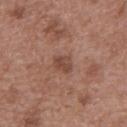This lesion was catalogued during total-body skin photography and was not selected for biopsy. A male patient aged around 50. Automated tile analysis of the lesion measured a footprint of about 4 mm², an outline eccentricity of about 0.6 (0 = round, 1 = elongated), and two-axis asymmetry of about 0.25. And it measured a lesion color around L≈46 a*≈21 b*≈27 in CIELAB and about 8 CIELAB-L* units darker than the surrounding skin. Captured under white-light illumination. Measured at roughly 2.5 mm in maximum diameter. On the mid back. A 15 mm close-up tile from a total-body photography series done for melanoma screening.